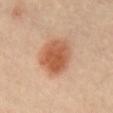Q: What is the imaging modality?
A: ~15 mm crop, total-body skin-cancer survey
Q: How was the tile lit?
A: cross-polarized illumination
Q: What is the lesion's diameter?
A: ≈5 mm
Q: Lesion location?
A: the front of the torso